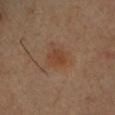Findings:
– biopsy status: no biopsy performed (imaged during a skin exam)
– site: the chest
– image: ~15 mm crop, total-body skin-cancer survey
– patient: male, in their mid- to late 30s